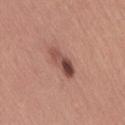Q: Was this lesion biopsied?
A: no biopsy performed (imaged during a skin exam)
Q: Lesion location?
A: the leg
Q: What kind of image is this?
A: ~15 mm tile from a whole-body skin photo
Q: What did automated image analysis measure?
A: a nevus-likeness score of about 70/100 and lesion-presence confidence of about 100/100
Q: How large is the lesion?
A: about 4.5 mm
Q: What are the patient's age and sex?
A: female, aged 28 to 32